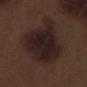Q: What is the anatomic site?
A: the left thigh
Q: Who is the patient?
A: male, about 70 years old
Q: What is the imaging modality?
A: ~15 mm crop, total-body skin-cancer survey